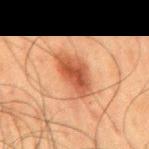On the mid back.
The tile uses cross-polarized illumination.
A male patient aged 58–62.
Longest diameter approximately 5.5 mm.
A lesion tile, about 15 mm wide, cut from a 3D total-body photograph.